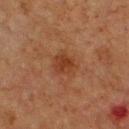The lesion was photographed on a routine skin check and not biopsied; there is no pathology result. Measured at roughly 3 mm in maximum diameter. Located on the chest. Captured under cross-polarized illumination. Automated tile analysis of the lesion measured a footprint of about 6.5 mm² and an eccentricity of roughly 0.5. The analysis additionally found a lesion color around L≈31 a*≈20 b*≈29 in CIELAB and a normalized border contrast of about 7. The software also gave a border-irregularity index near 2.5/10, a within-lesion color-variation index near 3/10, and radial color variation of about 1. A 15 mm close-up extracted from a 3D total-body photography capture. A male subject aged around 75.